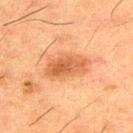  image:
    source: total-body photography crop
    field_of_view_mm: 15
  site: upper back
  patient:
    sex: male
    age_approx: 50
  automated_metrics:
    cielab_L: 48
    cielab_a: 23
    cielab_b: 34
    vs_skin_contrast_norm: 7.5
  lighting: cross-polarized